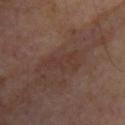This lesion was catalogued during total-body skin photography and was not selected for biopsy. The tile uses cross-polarized illumination. The lesion is located on the upper back. An algorithmic analysis of the crop reported a border-irregularity index near 7.5/10, a color-variation rating of about 1/10, and a peripheral color-asymmetry measure near 0.5. This image is a 15 mm lesion crop taken from a total-body photograph. A subject aged around 55. The lesion's longest dimension is about 5 mm.